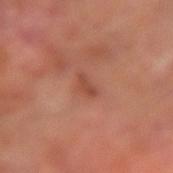No biopsy was performed on this lesion — it was imaged during a full skin examination and was not determined to be concerning.